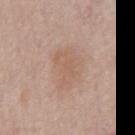Part of a total-body skin-imaging series; this lesion was reviewed on a skin check and was not flagged for biopsy. The recorded lesion diameter is about 4.5 mm. The patient is a male aged approximately 60. A roughly 15 mm field-of-view crop from a total-body skin photograph. This is a white-light tile. From the chest.